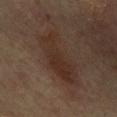Part of a total-body skin-imaging series; this lesion was reviewed on a skin check and was not flagged for biopsy. The recorded lesion diameter is about 8 mm. The tile uses cross-polarized illumination. An algorithmic analysis of the crop reported a footprint of about 18 mm² and an outline eccentricity of about 0.95 (0 = round, 1 = elongated). The software also gave about 6 CIELAB-L* units darker than the surrounding skin. The software also gave border irregularity of about 5.5 on a 0–10 scale, a color-variation rating of about 3/10, and radial color variation of about 1. A male subject in their mid- to late 60s. Located on the chest. A roughly 15 mm field-of-view crop from a total-body skin photograph.